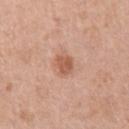Assessment:
The lesion was tiled from a total-body skin photograph and was not biopsied.
Context:
A female patient aged 43 to 47. Located on the right upper arm. A region of skin cropped from a whole-body photographic capture, roughly 15 mm wide. This is a white-light tile.